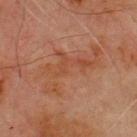follow-up=catalogued during a skin exam; not biopsied
image=~15 mm crop, total-body skin-cancer survey
TBP lesion metrics=a lesion area of about 19 mm², an eccentricity of roughly 0.9, and two-axis asymmetry of about 0.6; a lesion color around L≈39 a*≈21 b*≈28 in CIELAB and roughly 5 lightness units darker than nearby skin; a border-irregularity index near 9.5/10, internal color variation of about 3.5 on a 0–10 scale, and peripheral color asymmetry of about 1; an automated nevus-likeness rating near 0 out of 100 and a lesion-detection confidence of about 100/100
illumination=cross-polarized
patient=male, aged 68–72
lesion diameter=≈8 mm
site=the chest